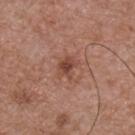| field | value |
|---|---|
| notes | total-body-photography surveillance lesion; no biopsy |
| anatomic site | the chest |
| lighting | white-light |
| imaging modality | 15 mm crop, total-body photography |
| subject | male, in their mid- to late 50s |